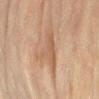Clinical impression:
No biopsy was performed on this lesion — it was imaged during a full skin examination and was not determined to be concerning.
Context:
On the left forearm. A roughly 15 mm field-of-view crop from a total-body skin photograph. Imaged with cross-polarized lighting. The patient is a female roughly 80 years of age. Automated image analysis of the tile measured a lesion area of about 9.5 mm². The software also gave an average lesion color of about L≈50 a*≈17 b*≈28 (CIELAB), about 7 CIELAB-L* units darker than the surrounding skin, and a normalized border contrast of about 5.5. The analysis additionally found border irregularity of about 6 on a 0–10 scale, a color-variation rating of about 2.5/10, and a peripheral color-asymmetry measure near 0.5. The analysis additionally found a nevus-likeness score of about 0/100.